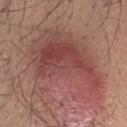Impression: Recorded during total-body skin imaging; not selected for excision or biopsy. Context: The lesion-visualizer software estimated a nevus-likeness score of about 100/100 and a detector confidence of about 100 out of 100 that the crop contains a lesion. A 15 mm close-up tile from a total-body photography series done for melanoma screening. On the head or neck. A male subject, about 30 years old. This is a white-light tile. Longest diameter approximately 12 mm.